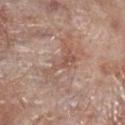* follow-up · imaged on a skin check; not biopsied
* patient · male, approximately 70 years of age
* lighting · white-light illumination
* image source · total-body-photography crop, ~15 mm field of view
* site · the right lower leg
* lesion diameter · ~5.5 mm (longest diameter)